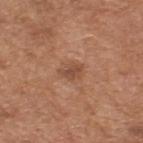Assessment:
Imaged during a routine full-body skin examination; the lesion was not biopsied and no histopathology is available.
Image and clinical context:
Captured under white-light illumination. The total-body-photography lesion software estimated a mean CIELAB color near L≈48 a*≈22 b*≈30. It also reported internal color variation of about 1.5 on a 0–10 scale and a peripheral color-asymmetry measure near 0.5. A male subject in their mid- to late 60s. This image is a 15 mm lesion crop taken from a total-body photograph. The lesion is on the upper back. About 2.5 mm across.